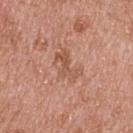No biopsy was performed on this lesion — it was imaged during a full skin examination and was not determined to be concerning. The subject is a male aged around 55. Imaged with white-light lighting. Cropped from a total-body skin-imaging series; the visible field is about 15 mm. Approximately 4 mm at its widest. Automated tile analysis of the lesion measured a footprint of about 6.5 mm², an eccentricity of roughly 0.85, and two-axis asymmetry of about 0.5. And it measured a lesion color around L≈54 a*≈23 b*≈31 in CIELAB and a lesion–skin lightness drop of about 8. And it measured a nevus-likeness score of about 0/100 and a lesion-detection confidence of about 100/100. From the back.